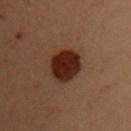| field | value |
|---|---|
| biopsy status | total-body-photography surveillance lesion; no biopsy |
| automated lesion analysis | an area of roughly 12 mm², an eccentricity of roughly 0.65, and a symmetry-axis asymmetry near 0.1; a lesion color around L≈20 a*≈18 b*≈22 in CIELAB, roughly 12 lightness units darker than nearby skin, and a normalized lesion–skin contrast near 14 |
| diameter | about 4.5 mm |
| image | ~15 mm crop, total-body skin-cancer survey |
| patient | female, approximately 40 years of age |
| anatomic site | the right upper arm |
| lighting | cross-polarized |